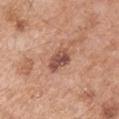{"biopsy_status": "not biopsied; imaged during a skin examination", "patient": {"sex": "male", "age_approx": 55}, "image": {"source": "total-body photography crop", "field_of_view_mm": 15}, "site": "right upper arm"}